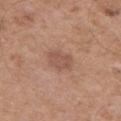Q: Was this lesion biopsied?
A: total-body-photography surveillance lesion; no biopsy
Q: How was the tile lit?
A: white-light illumination
Q: Lesion location?
A: the right upper arm
Q: How large is the lesion?
A: ≈2.5 mm
Q: Who is the patient?
A: male, aged approximately 50
Q: How was this image acquired?
A: ~15 mm crop, total-body skin-cancer survey